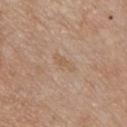follow-up = no biopsy performed (imaged during a skin exam); acquisition = ~15 mm tile from a whole-body skin photo; tile lighting = white-light; location = the chest; patient = male, approximately 55 years of age.